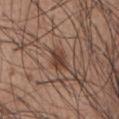| key | value |
|---|---|
| lesion diameter | about 3 mm |
| subject | male, aged around 55 |
| lighting | white-light |
| location | the abdomen |
| image source | total-body-photography crop, ~15 mm field of view |